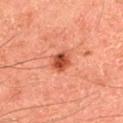Clinical impression:
The lesion was photographed on a routine skin check and not biopsied; there is no pathology result.
Background:
The patient is a male roughly 45 years of age. The total-body-photography lesion software estimated an area of roughly 5.5 mm², an outline eccentricity of about 0.6 (0 = round, 1 = elongated), and a shape-asymmetry score of about 0.2 (0 = symmetric). The analysis additionally found a lesion color around L≈50 a*≈33 b*≈37 in CIELAB and a lesion–skin lightness drop of about 14. It also reported a border-irregularity index near 1.5/10, a within-lesion color-variation index near 5/10, and radial color variation of about 2. It also reported an automated nevus-likeness rating near 100 out of 100 and lesion-presence confidence of about 100/100. On the upper back. The tile uses cross-polarized illumination. About 3 mm across. Cropped from a whole-body photographic skin survey; the tile spans about 15 mm.